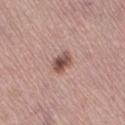Findings:
* notes: no biopsy performed (imaged during a skin exam)
* image source: 15 mm crop, total-body photography
* body site: the right thigh
* patient: female, roughly 60 years of age
* illumination: white-light
* lesion size: ~3 mm (longest diameter)